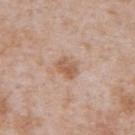  biopsy_status: not biopsied; imaged during a skin examination
  lighting: white-light
  lesion_size:
    long_diameter_mm_approx: 3.0
  patient:
    sex: male
    age_approx: 65
  image:
    source: total-body photography crop
    field_of_view_mm: 15
  automated_metrics:
    cielab_L: 58
    cielab_a: 20
    cielab_b: 31
    vs_skin_darker_L: 9.0
    vs_skin_contrast_norm: 7.0
    border_irregularity_0_10: 2.0
    color_variation_0_10: 4.0
    peripheral_color_asymmetry: 1.5
    lesion_detection_confidence_0_100: 100
  site: abdomen